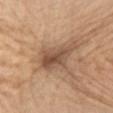biopsy_status: not biopsied; imaged during a skin examination
image:
  source: total-body photography crop
  field_of_view_mm: 15
site: abdomen
automated_metrics:
  area_mm2_approx: 14.0
  eccentricity: 0.8
  shape_asymmetry: 0.4
  vs_skin_contrast_norm: 7.5
  nevus_likeness_0_100: 20
  lesion_detection_confidence_0_100: 80
lighting: white-light
patient:
  sex: female
  age_approx: 70
lesion_size:
  long_diameter_mm_approx: 5.5